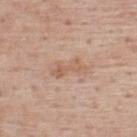  biopsy_status: not biopsied; imaged during a skin examination
  patient:
    sex: male
    age_approx: 75
  image:
    source: total-body photography crop
    field_of_view_mm: 15
  site: upper back